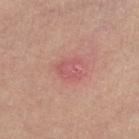Q: Was a biopsy performed?
A: imaged on a skin check; not biopsied
Q: What did automated image analysis measure?
A: a border-irregularity index near 3.5/10, internal color variation of about 1.5 on a 0–10 scale, and peripheral color asymmetry of about 0.5; a classifier nevus-likeness of about 0/100 and a lesion-detection confidence of about 100/100
Q: Lesion size?
A: about 3 mm
Q: What is the imaging modality?
A: ~15 mm crop, total-body skin-cancer survey
Q: Lesion location?
A: the left lower leg
Q: What lighting was used for the tile?
A: white-light illumination
Q: Patient demographics?
A: female, roughly 65 years of age